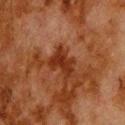The lesion was photographed on a routine skin check and not biopsied; there is no pathology result.